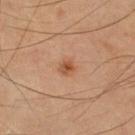notes = no biopsy performed (imaged during a skin exam)
lesion size = about 2 mm
lighting = cross-polarized
image = ~15 mm crop, total-body skin-cancer survey
location = the right thigh
subject = male, about 65 years old
automated metrics = an area of roughly 3 mm² and an eccentricity of roughly 0.3; about 10 CIELAB-L* units darker than the surrounding skin; border irregularity of about 2.5 on a 0–10 scale, a color-variation rating of about 3.5/10, and peripheral color asymmetry of about 1; an automated nevus-likeness rating near 85 out of 100 and a lesion-detection confidence of about 100/100